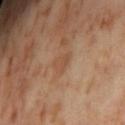Assessment: The lesion was tiled from a total-body skin photograph and was not biopsied. Image and clinical context: A lesion tile, about 15 mm wide, cut from a 3D total-body photograph. The tile uses cross-polarized illumination. A female patient, approximately 55 years of age. An algorithmic analysis of the crop reported a footprint of about 4.5 mm² and a symmetry-axis asymmetry near 0.35. The software also gave an average lesion color of about L≈51 a*≈20 b*≈32 (CIELAB), a lesion–skin lightness drop of about 6, and a normalized border contrast of about 5. The analysis additionally found a border-irregularity rating of about 3/10 and a color-variation rating of about 2/10. And it measured an automated nevus-likeness rating near 0 out of 100 and a detector confidence of about 100 out of 100 that the crop contains a lesion. Longest diameter approximately 3 mm. The lesion is located on the left thigh.